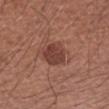- biopsy status — catalogued during a skin exam; not biopsied
- imaging modality — ~15 mm crop, total-body skin-cancer survey
- tile lighting — white-light illumination
- size — ~3.5 mm (longest diameter)
- patient — male, aged around 65
- site — the left forearm